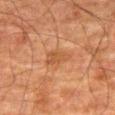Q: Was this lesion biopsied?
A: catalogued during a skin exam; not biopsied
Q: Lesion size?
A: ~3.5 mm (longest diameter)
Q: How was the tile lit?
A: cross-polarized illumination
Q: What is the anatomic site?
A: the right thigh
Q: What kind of image is this?
A: ~15 mm crop, total-body skin-cancer survey
Q: What are the patient's age and sex?
A: male, aged 78 to 82